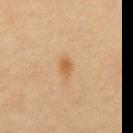Background:
Measured at roughly 2.5 mm in maximum diameter. This is a cross-polarized tile. A male subject roughly 60 years of age. The total-body-photography lesion software estimated a lesion area of about 3 mm² and a shape eccentricity near 0.75. And it measured roughly 9 lightness units darker than nearby skin and a lesion-to-skin contrast of about 7 (normalized; higher = more distinct). The software also gave border irregularity of about 2 on a 0–10 scale, a within-lesion color-variation index near 2.5/10, and radial color variation of about 1. On the abdomen. A 15 mm close-up extracted from a 3D total-body photography capture.